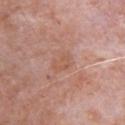Findings:
- notes: catalogued during a skin exam; not biopsied
- diameter: ~3 mm (longest diameter)
- subject: male, approximately 80 years of age
- site: the chest
- illumination: white-light illumination
- image source: 15 mm crop, total-body photography